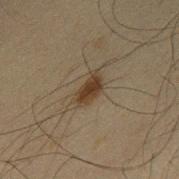Q: Is there a histopathology result?
A: imaged on a skin check; not biopsied
Q: Illumination type?
A: cross-polarized illumination
Q: What is the imaging modality?
A: ~15 mm crop, total-body skin-cancer survey
Q: What is the anatomic site?
A: the mid back
Q: What is the lesion's diameter?
A: about 3.5 mm
Q: What are the patient's age and sex?
A: male, aged approximately 65
Q: Automated lesion metrics?
A: border irregularity of about 3.5 on a 0–10 scale, a color-variation rating of about 2/10, and a peripheral color-asymmetry measure near 0.5; a detector confidence of about 100 out of 100 that the crop contains a lesion